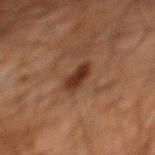Context:
Cropped from a total-body skin-imaging series; the visible field is about 15 mm. This is a cross-polarized tile. The recorded lesion diameter is about 3.5 mm. The lesion is on the back. The patient is a male aged 58 to 62.